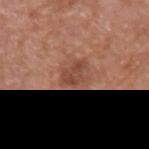Captured during whole-body skin photography for melanoma surveillance; the lesion was not biopsied. A 15 mm close-up extracted from a 3D total-body photography capture. From the left upper arm. The patient is a male approximately 65 years of age.